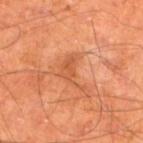{"biopsy_status": "not biopsied; imaged during a skin examination", "patient": {"sex": "male", "age_approx": 65}, "automated_metrics": {"area_mm2_approx": 7.0, "eccentricity": 0.8, "shape_asymmetry": 0.65, "vs_skin_darker_L": 7.0, "border_irregularity_0_10": 8.0, "peripheral_color_asymmetry": 0.5}, "image": {"source": "total-body photography crop", "field_of_view_mm": 15}, "lighting": "cross-polarized", "site": "left lower leg", "lesion_size": {"long_diameter_mm_approx": 4.5}}